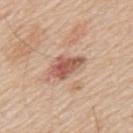{
  "biopsy_status": "not biopsied; imaged during a skin examination",
  "site": "mid back",
  "patient": {
    "sex": "male",
    "age_approx": 60
  },
  "image": {
    "source": "total-body photography crop",
    "field_of_view_mm": 15
  }
}